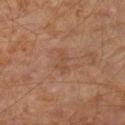<record>
<biopsy_status>not biopsied; imaged during a skin examination</biopsy_status>
<lighting>cross-polarized</lighting>
<image>
  <source>total-body photography crop</source>
  <field_of_view_mm>15</field_of_view_mm>
</image>
<lesion_size>
  <long_diameter_mm_approx>3.0</long_diameter_mm_approx>
</lesion_size>
<patient>
  <sex>male</sex>
  <age_approx>60</age_approx>
</patient>
<site>left lower leg</site>
</record>